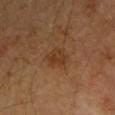notes = imaged on a skin check; not biopsied
tile lighting = cross-polarized illumination
imaging modality = total-body-photography crop, ~15 mm field of view
subject = male, in their 60s
lesion size = about 3 mm
site = the right upper arm